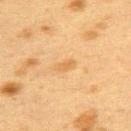Assessment:
Recorded during total-body skin imaging; not selected for excision or biopsy.
Clinical summary:
Cropped from a whole-body photographic skin survey; the tile spans about 15 mm. Approximately 2.5 mm at its widest. From the upper back. A female patient, aged approximately 40. The total-body-photography lesion software estimated an area of roughly 3 mm². The tile uses cross-polarized illumination.